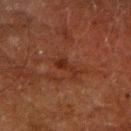Q: Is there a histopathology result?
A: no biopsy performed (imaged during a skin exam)
Q: Lesion location?
A: the arm
Q: What did automated image analysis measure?
A: a lesion color around L≈22 a*≈20 b*≈25 in CIELAB and a lesion–skin lightness drop of about 6; a nevus-likeness score of about 0/100 and a lesion-detection confidence of about 100/100
Q: How was this image acquired?
A: ~15 mm crop, total-body skin-cancer survey
Q: What are the patient's age and sex?
A: male, aged 58–62